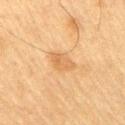patient:
  sex: male
  age_approx: 70
automated_metrics:
  area_mm2_approx: 5.0
  eccentricity: 0.7
  shape_asymmetry: 0.3
  cielab_L: 54
  cielab_a: 18
  cielab_b: 37
  vs_skin_darker_L: 7.0
  vs_skin_contrast_norm: 5.5
  border_irregularity_0_10: 3.0
  peripheral_color_asymmetry: 1.0
lighting: cross-polarized
image:
  source: total-body photography crop
  field_of_view_mm: 15
site: right upper arm
lesion_size:
  long_diameter_mm_approx: 3.0